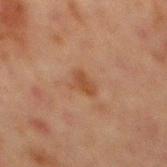workup = no biopsy performed (imaged during a skin exam)
illumination = cross-polarized illumination
diameter = ~3 mm (longest diameter)
image = total-body-photography crop, ~15 mm field of view
anatomic site = the abdomen
automated lesion analysis = border irregularity of about 4 on a 0–10 scale, a color-variation rating of about 2/10, and radial color variation of about 0.5
patient = male, aged approximately 65